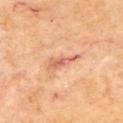Notes:
• biopsy status: total-body-photography surveillance lesion; no biopsy
• size: ≈4 mm
• tile lighting: cross-polarized illumination
• location: the upper back
• automated lesion analysis: a mean CIELAB color near L≈62 a*≈27 b*≈33, about 10 CIELAB-L* units darker than the surrounding skin, and a normalized lesion–skin contrast near 6.5; border irregularity of about 5.5 on a 0–10 scale, a color-variation rating of about 3/10, and peripheral color asymmetry of about 0.5
• patient: male, aged around 70
• image: total-body-photography crop, ~15 mm field of view